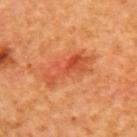<tbp_lesion>
<biopsy_status>not biopsied; imaged during a skin examination</biopsy_status>
<image>
  <source>total-body photography crop</source>
  <field_of_view_mm>15</field_of_view_mm>
</image>
<patient>
  <sex>female</sex>
  <age_approx>40</age_approx>
</patient>
<site>upper back</site>
</tbp_lesion>